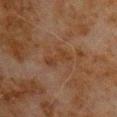Q: Was this lesion biopsied?
A: imaged on a skin check; not biopsied
Q: How was the tile lit?
A: cross-polarized
Q: What kind of image is this?
A: 15 mm crop, total-body photography
Q: Who is the patient?
A: male, in their 80s
Q: Where on the body is the lesion?
A: the upper back